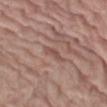Assessment: No biopsy was performed on this lesion — it was imaged during a full skin examination and was not determined to be concerning. Background: Measured at roughly 3 mm in maximum diameter. A male patient, in their 80s. The tile uses white-light illumination. A close-up tile cropped from a whole-body skin photograph, about 15 mm across. Located on the left thigh.